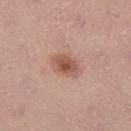<lesion>
<biopsy_status>not biopsied; imaged during a skin examination</biopsy_status>
<image>
  <source>total-body photography crop</source>
  <field_of_view_mm>15</field_of_view_mm>
</image>
<lesion_size>
  <long_diameter_mm_approx>3.5</long_diameter_mm_approx>
</lesion_size>
<lighting>white-light</lighting>
<site>right lower leg</site>
<automated_metrics>
  <area_mm2_approx>7.0</area_mm2_approx>
  <shape_asymmetry>0.2</shape_asymmetry>
  <cielab_L>54</cielab_L>
  <cielab_a>22</cielab_a>
  <cielab_b>27</cielab_b>
  <vs_skin_darker_L>11.0</vs_skin_darker_L>
  <vs_skin_contrast_norm>7.5</vs_skin_contrast_norm>
</automated_metrics>
<patient>
  <sex>male</sex>
  <age_approx>35</age_approx>
</patient>
</lesion>